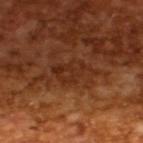Impression:
The lesion was photographed on a routine skin check and not biopsied; there is no pathology result.
Context:
A roughly 15 mm field-of-view crop from a total-body skin photograph. The lesion's longest dimension is about 5 mm. The patient is a male approximately 65 years of age. Imaged with cross-polarized lighting.